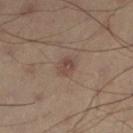biopsy status: no biopsy performed (imaged during a skin exam)
image: 15 mm crop, total-body photography
patient: male, about 55 years old
body site: the left lower leg